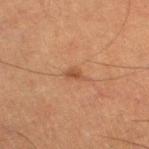Assessment: Captured during whole-body skin photography for melanoma surveillance; the lesion was not biopsied. Image and clinical context: The tile uses cross-polarized illumination. Located on the left thigh. A 15 mm close-up extracted from a 3D total-body photography capture. A female patient, approximately 60 years of age. Measured at roughly 2.5 mm in maximum diameter. An algorithmic analysis of the crop reported a lesion area of about 3 mm², an eccentricity of roughly 0.8, and a shape-asymmetry score of about 0.35 (0 = symmetric). And it measured a border-irregularity index near 3.5/10 and a peripheral color-asymmetry measure near 0.5. The analysis additionally found a nevus-likeness score of about 10/100.